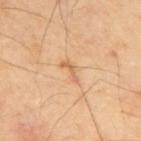| key | value |
|---|---|
| follow-up | no biopsy performed (imaged during a skin exam) |
| location | the upper back |
| size | ≈3 mm |
| illumination | cross-polarized illumination |
| image | 15 mm crop, total-body photography |
| subject | male, in their 40s |